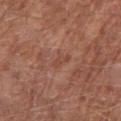Acquisition and patient details: The lesion is located on the right thigh. An algorithmic analysis of the crop reported a footprint of about 2.5 mm², a shape eccentricity near 0.85, and a shape-asymmetry score of about 0.5 (0 = symmetric). And it measured a classifier nevus-likeness of about 0/100 and a lesion-detection confidence of about 100/100. The tile uses white-light illumination. Longest diameter approximately 2.5 mm. This image is a 15 mm lesion crop taken from a total-body photograph. A male patient about 65 years old.